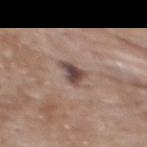Q: Is there a histopathology result?
A: catalogued during a skin exam; not biopsied
Q: Illumination type?
A: white-light
Q: Patient demographics?
A: female, aged 58–62
Q: Lesion size?
A: ≈3 mm
Q: What is the imaging modality?
A: total-body-photography crop, ~15 mm field of view
Q: What did automated image analysis measure?
A: an area of roughly 4 mm², a shape eccentricity near 0.65, and a symmetry-axis asymmetry near 0.45; a lesion-to-skin contrast of about 11 (normalized; higher = more distinct)
Q: Where on the body is the lesion?
A: the upper back